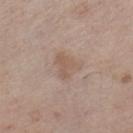The lesion was photographed on a routine skin check and not biopsied; there is no pathology result.
Approximately 3.5 mm at its widest.
On the right lower leg.
The lesion-visualizer software estimated two-axis asymmetry of about 0.45. And it measured a border-irregularity rating of about 4.5/10, internal color variation of about 2 on a 0–10 scale, and radial color variation of about 0.5.
This is a white-light tile.
Cropped from a total-body skin-imaging series; the visible field is about 15 mm.
The patient is a female approximately 55 years of age.